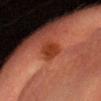notes: total-body-photography surveillance lesion; no biopsy
location: the head or neck
subject: male, aged around 35
image source: 15 mm crop, total-body photography
lighting: cross-polarized illumination